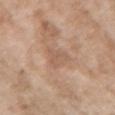Recorded during total-body skin imaging; not selected for excision or biopsy. The subject is a female approximately 75 years of age. Cropped from a whole-body photographic skin survey; the tile spans about 15 mm. The lesion is on the left forearm.